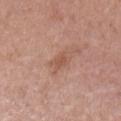Imaged during a routine full-body skin examination; the lesion was not biopsied and no histopathology is available. Longest diameter approximately 3 mm. A female patient aged 33–37. A lesion tile, about 15 mm wide, cut from a 3D total-body photograph. Imaged with white-light lighting. On the left upper arm. An algorithmic analysis of the crop reported a lesion area of about 3 mm². And it measured a mean CIELAB color near L≈54 a*≈23 b*≈29, a lesion–skin lightness drop of about 7, and a lesion-to-skin contrast of about 5.5 (normalized; higher = more distinct). It also reported internal color variation of about 1 on a 0–10 scale and peripheral color asymmetry of about 0.5. It also reported a classifier nevus-likeness of about 0/100 and a detector confidence of about 100 out of 100 that the crop contains a lesion.